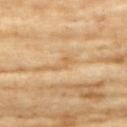Recorded during total-body skin imaging; not selected for excision or biopsy.
A close-up tile cropped from a whole-body skin photograph, about 15 mm across.
The tile uses cross-polarized illumination.
Automated tile analysis of the lesion measured an area of roughly 3 mm² and two-axis asymmetry of about 0.45. The software also gave an average lesion color of about L≈64 a*≈18 b*≈41 (CIELAB), roughly 7 lightness units darker than nearby skin, and a lesion-to-skin contrast of about 5 (normalized; higher = more distinct). The analysis additionally found a border-irregularity rating of about 5/10 and a within-lesion color-variation index near 0/10. It also reported a nevus-likeness score of about 0/100 and a detector confidence of about 95 out of 100 that the crop contains a lesion.
Approximately 3 mm at its widest.
The subject is a female approximately 75 years of age.
On the chest.